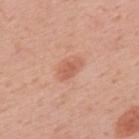Impression:
The lesion was tiled from a total-body skin photograph and was not biopsied.
Acquisition and patient details:
A male patient, aged 58–62. Located on the back. The tile uses white-light illumination. Cropped from a total-body skin-imaging series; the visible field is about 15 mm. Automated tile analysis of the lesion measured an eccentricity of roughly 0.85 and a shape-asymmetry score of about 0.3 (0 = symmetric). It also reported an average lesion color of about L≈59 a*≈27 b*≈32 (CIELAB), roughly 9 lightness units darker than nearby skin, and a lesion-to-skin contrast of about 6 (normalized; higher = more distinct). It also reported a peripheral color-asymmetry measure near 0.5. It also reported a nevus-likeness score of about 65/100 and a lesion-detection confidence of about 100/100.